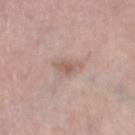Q: Was this lesion biopsied?
A: total-body-photography surveillance lesion; no biopsy
Q: How large is the lesion?
A: ~3 mm (longest diameter)
Q: Automated lesion metrics?
A: an area of roughly 3.5 mm² and an eccentricity of roughly 0.9; a lesion color around L≈58 a*≈17 b*≈25 in CIELAB, a lesion–skin lightness drop of about 9, and a normalized lesion–skin contrast near 6.5; a classifier nevus-likeness of about 0/100 and a detector confidence of about 100 out of 100 that the crop contains a lesion
Q: How was the tile lit?
A: white-light illumination
Q: Lesion location?
A: the left lower leg
Q: Patient demographics?
A: female, aged 63 to 67
Q: What is the imaging modality?
A: ~15 mm crop, total-body skin-cancer survey